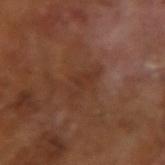Imaged during a routine full-body skin examination; the lesion was not biopsied and no histopathology is available. A 15 mm close-up tile from a total-body photography series done for melanoma screening. A male patient, roughly 65 years of age. Imaged with cross-polarized lighting. Longest diameter approximately 3.5 mm.